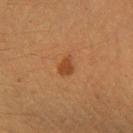biopsy_status: not biopsied; imaged during a skin examination
lighting: cross-polarized
lesion_size:
  long_diameter_mm_approx: 2.5
site: left forearm
automated_metrics:
  area_mm2_approx: 3.5
  shape_asymmetry: 0.2
  cielab_L: 39
  cielab_a: 22
  cielab_b: 35
  vs_skin_darker_L: 8.0
image:
  source: total-body photography crop
  field_of_view_mm: 15
patient:
  sex: female
  age_approx: 40